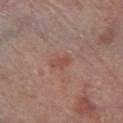No biopsy was performed on this lesion — it was imaged during a full skin examination and was not determined to be concerning.
Captured under white-light illumination.
The lesion is on the right lower leg.
Automated tile analysis of the lesion measured a lesion area of about 3 mm², an outline eccentricity of about 0.85 (0 = round, 1 = elongated), and a symmetry-axis asymmetry near 0.35. It also reported a lesion color around L≈49 a*≈23 b*≈25 in CIELAB, a lesion–skin lightness drop of about 7, and a normalized border contrast of about 5.5. It also reported a classifier nevus-likeness of about 0/100.
A 15 mm close-up extracted from a 3D total-body photography capture.
The subject is a male in their 70s.
Approximately 2.5 mm at its widest.